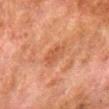biopsy_status: not biopsied; imaged during a skin examination
automated_metrics:
  area_mm2_approx: 4.5
  eccentricity: 0.8
  vs_skin_darker_L: 6.0
  color_variation_0_10: 1.5
  peripheral_color_asymmetry: 0.5
lesion_size:
  long_diameter_mm_approx: 3.0
lighting: cross-polarized
image:
  source: total-body photography crop
  field_of_view_mm: 15
patient:
  sex: male
  age_approx: 80
site: right lower leg